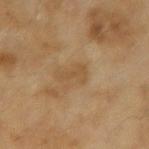Captured during whole-body skin photography for melanoma surveillance; the lesion was not biopsied. Automated image analysis of the tile measured an eccentricity of roughly 0.9 and a symmetry-axis asymmetry near 0.6. It also reported a lesion color around L≈46 a*≈15 b*≈34 in CIELAB, a lesion–skin lightness drop of about 5, and a lesion-to-skin contrast of about 5 (normalized; higher = more distinct). The software also gave lesion-presence confidence of about 100/100. About 3.5 mm across. This is a cross-polarized tile. From the arm. Cropped from a total-body skin-imaging series; the visible field is about 15 mm. A female subject aged approximately 60.